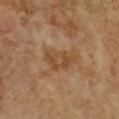| feature | finding |
|---|---|
| follow-up | no biopsy performed (imaged during a skin exam) |
| automated metrics | a shape-asymmetry score of about 0.35 (0 = symmetric); border irregularity of about 3.5 on a 0–10 scale, a within-lesion color-variation index near 3/10, and peripheral color asymmetry of about 1 |
| size | about 4.5 mm |
| subject | female, aged 58 to 62 |
| image source | 15 mm crop, total-body photography |
| lighting | cross-polarized |
| location | the chest |